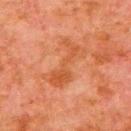Assessment: No biopsy was performed on this lesion — it was imaged during a full skin examination and was not determined to be concerning. Acquisition and patient details: A 15 mm close-up extracted from a 3D total-body photography capture. The patient is a male aged 78 to 82. The lesion is on the arm.